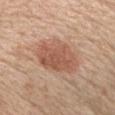Cropped from a whole-body photographic skin survey; the tile spans about 15 mm.
Captured under white-light illumination.
Automated tile analysis of the lesion measured a lesion color around L≈55 a*≈23 b*≈31 in CIELAB and a lesion–skin lightness drop of about 10. And it measured a border-irregularity rating of about 2.5/10, a within-lesion color-variation index near 3/10, and a peripheral color-asymmetry measure near 1.
On the head or neck.
A male patient aged around 60.